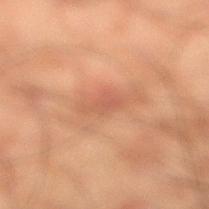follow-up = total-body-photography surveillance lesion; no biopsy | image source = 15 mm crop, total-body photography | tile lighting = cross-polarized illumination | anatomic site = the right lower leg | patient = male, in their 50s | lesion diameter = about 3 mm.